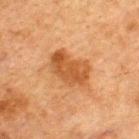Clinical impression: The lesion was photographed on a routine skin check and not biopsied; there is no pathology result. Background: A male patient aged around 50. From the upper back. The total-body-photography lesion software estimated an average lesion color of about L≈43 a*≈22 b*≈36 (CIELAB), roughly 9 lightness units darker than nearby skin, and a normalized lesion–skin contrast near 8. A 15 mm close-up tile from a total-body photography series done for melanoma screening. Approximately 5 mm at its widest.